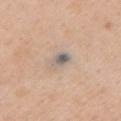size: ~2.5 mm (longest diameter)
body site: the chest
image: ~15 mm tile from a whole-body skin photo
subject: female, aged around 55